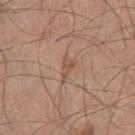Measured at roughly 3 mm in maximum diameter. A lesion tile, about 15 mm wide, cut from a 3D total-body photograph. Automated image analysis of the tile measured a footprint of about 3.5 mm² and an outline eccentricity of about 0.85 (0 = round, 1 = elongated). And it measured a mean CIELAB color near L≈53 a*≈19 b*≈29 and roughly 7 lightness units darker than nearby skin. The tile uses white-light illumination. A male patient, aged 43 to 47. The lesion is located on the left thigh.